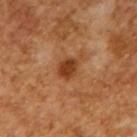This lesion was catalogued during total-body skin photography and was not selected for biopsy.
Longest diameter approximately 2.5 mm.
A close-up tile cropped from a whole-body skin photograph, about 15 mm across.
A male patient, in their mid-60s.